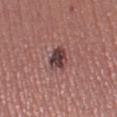Clinical impression: The lesion was tiled from a total-body skin photograph and was not biopsied. Acquisition and patient details: The lesion is located on the left thigh. The subject is a female roughly 30 years of age. A lesion tile, about 15 mm wide, cut from a 3D total-body photograph. Longest diameter approximately 3 mm.